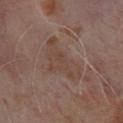notes = imaged on a skin check; not biopsied
automated lesion analysis = a footprint of about 14 mm² and a shape eccentricity near 0.8; an average lesion color of about L≈41 a*≈16 b*≈23 (CIELAB) and a normalized lesion–skin contrast near 5; an automated nevus-likeness rating near 0 out of 100 and lesion-presence confidence of about 100/100
size = ~6 mm (longest diameter)
subject = male, in their mid-60s
image source = 15 mm crop, total-body photography
illumination = cross-polarized illumination
anatomic site = the upper back